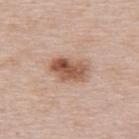Q: Was this lesion biopsied?
A: imaged on a skin check; not biopsied
Q: What lighting was used for the tile?
A: white-light illumination
Q: Patient demographics?
A: female, aged approximately 65
Q: What is the imaging modality?
A: total-body-photography crop, ~15 mm field of view
Q: Lesion location?
A: the upper back
Q: What did automated image analysis measure?
A: a border-irregularity index near 2.5/10 and a peripheral color-asymmetry measure near 3
Q: Lesion size?
A: ≈4.5 mm